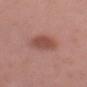  biopsy_status: not biopsied; imaged during a skin examination